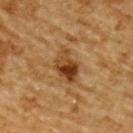The lesion was tiled from a total-body skin photograph and was not biopsied.
A 15 mm close-up extracted from a 3D total-body photography capture.
From the back.
Approximately 4.5 mm at its widest.
This is a cross-polarized tile.
An algorithmic analysis of the crop reported a footprint of about 9 mm², an eccentricity of roughly 0.75, and a shape-asymmetry score of about 0.25 (0 = symmetric). And it measured a classifier nevus-likeness of about 85/100 and lesion-presence confidence of about 100/100.
A male subject aged approximately 85 or older.